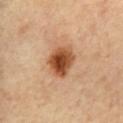lesion diameter = ≈4 mm | lighting = cross-polarized illumination | automated lesion analysis = a border-irregularity rating of about 2/10, a color-variation rating of about 7/10, and peripheral color asymmetry of about 2.5 | site = the chest | patient = female, roughly 35 years of age | image = 15 mm crop, total-body photography.